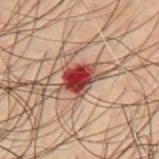Recorded during total-body skin imaging; not selected for excision or biopsy. A male subject, aged 48–52. On the mid back. A 15 mm close-up extracted from a 3D total-body photography capture.